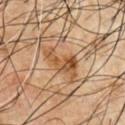This lesion was catalogued during total-body skin photography and was not selected for biopsy.
Cropped from a total-body skin-imaging series; the visible field is about 15 mm.
The lesion is on the front of the torso.
Captured under cross-polarized illumination.
Longest diameter approximately 5 mm.
A male subject aged 58 to 62.